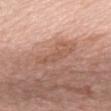Imaged during a routine full-body skin examination; the lesion was not biopsied and no histopathology is available. The lesion is on the mid back. A 15 mm close-up tile from a total-body photography series done for melanoma screening. A female patient, about 65 years old. Imaged with white-light lighting. Automated tile analysis of the lesion measured a footprint of about 7.5 mm², a shape eccentricity near 0.85, and two-axis asymmetry of about 0.5. It also reported a detector confidence of about 95 out of 100 that the crop contains a lesion.